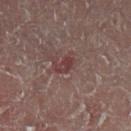The lesion was tiled from a total-body skin photograph and was not biopsied.